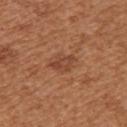The lesion was tiled from a total-body skin photograph and was not biopsied. An algorithmic analysis of the crop reported a footprint of about 4.5 mm² and two-axis asymmetry of about 0.25. The analysis additionally found border irregularity of about 3 on a 0–10 scale, a within-lesion color-variation index near 3/10, and peripheral color asymmetry of about 1. It also reported a classifier nevus-likeness of about 0/100. A female subject, aged approximately 40. On the upper back. About 3 mm across. Imaged with white-light lighting. A 15 mm close-up tile from a total-body photography series done for melanoma screening.